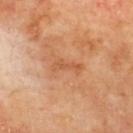Case summary:
• workup: total-body-photography surveillance lesion; no biopsy
• image source: total-body-photography crop, ~15 mm field of view
• site: the back
• illumination: cross-polarized illumination
• patient: male, approximately 70 years of age